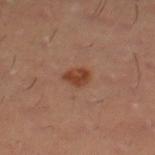Case summary:
• biopsy status · total-body-photography surveillance lesion; no biopsy
• site · the right lower leg
• image source · total-body-photography crop, ~15 mm field of view
• lighting · cross-polarized illumination
• lesion diameter · ≈2.5 mm
• patient · male, aged approximately 30